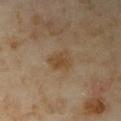Automated image analysis of the tile measured an area of roughly 5.5 mm², an eccentricity of roughly 0.7, and a shape-asymmetry score of about 0.25 (0 = symmetric). It also reported an average lesion color of about L≈43 a*≈15 b*≈32 (CIELAB), about 7 CIELAB-L* units darker than the surrounding skin, and a normalized lesion–skin contrast near 7. A 15 mm close-up extracted from a 3D total-body photography capture. This is a cross-polarized tile. The subject is a female about 35 years old. From the right upper arm.